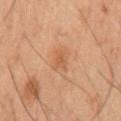| field | value |
|---|---|
| biopsy status | no biopsy performed (imaged during a skin exam) |
| size | about 2.5 mm |
| body site | the mid back |
| imaging modality | ~15 mm crop, total-body skin-cancer survey |
| subject | male, approximately 60 years of age |
| illumination | cross-polarized illumination |